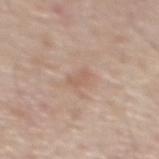Recorded during total-body skin imaging; not selected for excision or biopsy.
This is a white-light tile.
The lesion's longest dimension is about 2.5 mm.
A close-up tile cropped from a whole-body skin photograph, about 15 mm across.
The patient is a male aged around 55.
From the mid back.